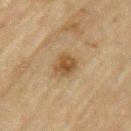The lesion was photographed on a routine skin check and not biopsied; there is no pathology result. A region of skin cropped from a whole-body photographic capture, roughly 15 mm wide. The patient is a male aged 68 to 72. The lesion is located on the left upper arm.